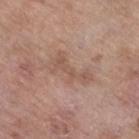workup: no biopsy performed (imaged during a skin exam).